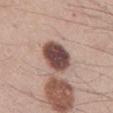Q: Was this lesion biopsied?
A: imaged on a skin check; not biopsied
Q: What lighting was used for the tile?
A: white-light illumination
Q: Patient demographics?
A: male, in their mid- to late 30s
Q: Where on the body is the lesion?
A: the mid back
Q: Lesion size?
A: ~5 mm (longest diameter)
Q: Automated lesion metrics?
A: a classifier nevus-likeness of about 30/100 and a detector confidence of about 100 out of 100 that the crop contains a lesion
Q: What kind of image is this?
A: ~15 mm crop, total-body skin-cancer survey